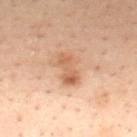{"biopsy_status": "not biopsied; imaged during a skin examination"}